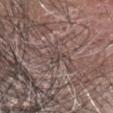notes: no biopsy performed (imaged during a skin exam) | lesion size: ≈2 mm | anatomic site: the head or neck | acquisition: ~15 mm crop, total-body skin-cancer survey | patient: male, aged around 45 | lighting: white-light.